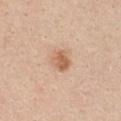This lesion was catalogued during total-body skin photography and was not selected for biopsy.
The subject is a female aged approximately 40.
A region of skin cropped from a whole-body photographic capture, roughly 15 mm wide.
From the chest.
The tile uses white-light illumination.